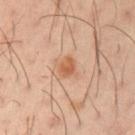follow-up: total-body-photography surveillance lesion; no biopsy
patient: male, aged 38 to 42
image: ~15 mm crop, total-body skin-cancer survey
site: the left upper arm